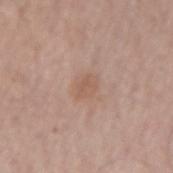Part of a total-body skin-imaging series; this lesion was reviewed on a skin check and was not flagged for biopsy.